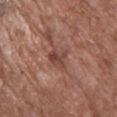notes: catalogued during a skin exam; not biopsied | patient: female, aged approximately 75 | lighting: white-light | acquisition: ~15 mm tile from a whole-body skin photo | lesion size: ≈3 mm | site: the upper back | TBP lesion metrics: an area of roughly 4.5 mm², an eccentricity of roughly 0.7, and two-axis asymmetry of about 0.35; an average lesion color of about L≈43 a*≈23 b*≈26 (CIELAB), roughly 9 lightness units darker than nearby skin, and a normalized lesion–skin contrast near 7.5; a border-irregularity index near 4.5/10, internal color variation of about 2 on a 0–10 scale, and a peripheral color-asymmetry measure near 0.5.